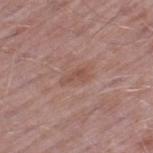| feature | finding |
|---|---|
| workup | catalogued during a skin exam; not biopsied |
| body site | the leg |
| size | about 3 mm |
| tile lighting | white-light |
| subject | male, aged around 50 |
| imaging modality | ~15 mm crop, total-body skin-cancer survey |